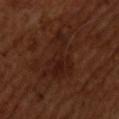  biopsy_status: not biopsied; imaged during a skin examination